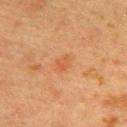Q: Who is the patient?
A: female, aged 53–57
Q: Illumination type?
A: cross-polarized
Q: What is the imaging modality?
A: total-body-photography crop, ~15 mm field of view
Q: Lesion location?
A: the upper back
Q: Automated lesion metrics?
A: a mean CIELAB color near L≈47 a*≈22 b*≈34 and a lesion–skin lightness drop of about 5
Q: How large is the lesion?
A: about 3 mm